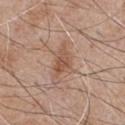The recorded lesion diameter is about 3.5 mm. This is a white-light tile. A 15 mm close-up extracted from a 3D total-body photography capture. The total-body-photography lesion software estimated internal color variation of about 3.5 on a 0–10 scale. The software also gave a nevus-likeness score of about 20/100 and a detector confidence of about 100 out of 100 that the crop contains a lesion. The lesion is located on the chest. A male subject, in their mid- to late 60s.